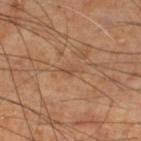No biopsy was performed on this lesion — it was imaged during a full skin examination and was not determined to be concerning. A 15 mm close-up extracted from a 3D total-body photography capture. The total-body-photography lesion software estimated an area of roughly 3 mm², an eccentricity of roughly 0.9, and a symmetry-axis asymmetry near 0.3. The tile uses cross-polarized illumination. The lesion is located on the leg. Measured at roughly 3 mm in maximum diameter. A male patient aged approximately 70.